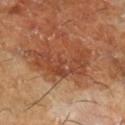Part of a total-body skin-imaging series; this lesion was reviewed on a skin check and was not flagged for biopsy.